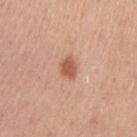{
  "image": {
    "source": "total-body photography crop",
    "field_of_view_mm": 15
  },
  "lighting": "white-light",
  "patient": {
    "sex": "female",
    "age_approx": 45
  },
  "site": "arm",
  "lesion_size": {
    "long_diameter_mm_approx": 2.5
  },
  "automated_metrics": {
    "area_mm2_approx": 4.0,
    "eccentricity": 0.7,
    "vs_skin_darker_L": 12.0,
    "vs_skin_contrast_norm": 8.0,
    "border_irregularity_0_10": 2.5,
    "color_variation_0_10": 1.5,
    "peripheral_color_asymmetry": 0.5
  }
}